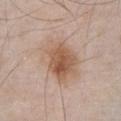Clinical impression:
This lesion was catalogued during total-body skin photography and was not selected for biopsy.
Context:
About 6 mm across. Captured under white-light illumination. Automated image analysis of the tile measured a lesion color around L≈58 a*≈18 b*≈29 in CIELAB, a lesion–skin lightness drop of about 10, and a normalized lesion–skin contrast near 7.5. And it measured a border-irregularity rating of about 2.5/10, a within-lesion color-variation index near 6.5/10, and radial color variation of about 2. It also reported an automated nevus-likeness rating near 90 out of 100. Cropped from a whole-body photographic skin survey; the tile spans about 15 mm. The subject is a male aged approximately 55. The lesion is located on the front of the torso.